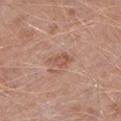subject: male, aged approximately 50 | tile lighting: white-light | automated metrics: a border-irregularity index near 2.5/10 and a within-lesion color-variation index near 2.5/10; an automated nevus-likeness rating near 20 out of 100 | body site: the left lower leg | acquisition: 15 mm crop, total-body photography.